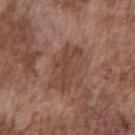Q: Was this lesion biopsied?
A: imaged on a skin check; not biopsied
Q: What are the patient's age and sex?
A: male, in their mid-70s
Q: How large is the lesion?
A: about 6 mm
Q: What kind of image is this?
A: total-body-photography crop, ~15 mm field of view
Q: Where on the body is the lesion?
A: the chest